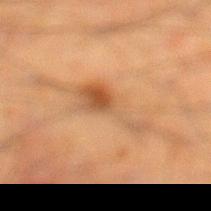biopsy status: catalogued during a skin exam; not biopsied | image: total-body-photography crop, ~15 mm field of view | automated lesion analysis: an outline eccentricity of about 0.95 (0 = round, 1 = elongated) and two-axis asymmetry of about 0.65; roughly 8 lightness units darker than nearby skin and a normalized lesion–skin contrast near 6.5; border irregularity of about 8.5 on a 0–10 scale and a peripheral color-asymmetry measure near 2 | subject: male, in their mid-30s | site: the left lower leg | tile lighting: cross-polarized illumination.